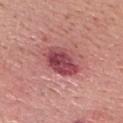Assessment: The lesion was tiled from a total-body skin photograph and was not biopsied. Image and clinical context: The patient is a male aged 63 to 67. A region of skin cropped from a whole-body photographic capture, roughly 15 mm wide. From the head or neck. Imaged with white-light lighting. Longest diameter approximately 5 mm.